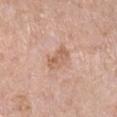Assessment: The lesion was tiled from a total-body skin photograph and was not biopsied. Background: A female patient approximately 70 years of age. A lesion tile, about 15 mm wide, cut from a 3D total-body photograph. Imaged with white-light lighting. On the leg. The total-body-photography lesion software estimated a footprint of about 5.5 mm², an outline eccentricity of about 0.8 (0 = round, 1 = elongated), and a shape-asymmetry score of about 0.25 (0 = symmetric). And it measured a normalized border contrast of about 6. The analysis additionally found border irregularity of about 2.5 on a 0–10 scale and a color-variation rating of about 3/10. The analysis additionally found a nevus-likeness score of about 0/100 and lesion-presence confidence of about 100/100. The lesion's longest dimension is about 3 mm.